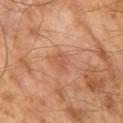Part of a total-body skin-imaging series; this lesion was reviewed on a skin check and was not flagged for biopsy.
A male subject, in their mid-60s.
This is a cross-polarized tile.
A close-up tile cropped from a whole-body skin photograph, about 15 mm across.
Automated tile analysis of the lesion measured an average lesion color of about L≈54 a*≈25 b*≈32 (CIELAB), about 6 CIELAB-L* units darker than the surrounding skin, and a normalized border contrast of about 4.5. And it measured a border-irregularity index near 4/10, a within-lesion color-variation index near 1/10, and a peripheral color-asymmetry measure near 0.5.
The recorded lesion diameter is about 2.5 mm.